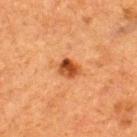{"biopsy_status": "not biopsied; imaged during a skin examination", "patient": {"sex": "male", "age_approx": 50}, "image": {"source": "total-body photography crop", "field_of_view_mm": 15}, "site": "upper back"}